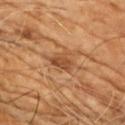lighting: cross-polarized
anatomic site: the chest
diameter: ≈3 mm
patient: male, aged 68–72
imaging modality: 15 mm crop, total-body photography
automated metrics: an outline eccentricity of about 0.8 (0 = round, 1 = elongated); a classifier nevus-likeness of about 15/100 and lesion-presence confidence of about 95/100